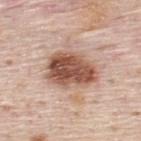biopsy status — total-body-photography surveillance lesion; no biopsy
subject — male, about 45 years old
body site — the upper back
acquisition — ~15 mm tile from a whole-body skin photo
lesion diameter — about 6 mm